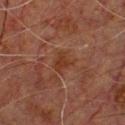Acquisition and patient details:
A male patient, aged around 50. From the chest. Imaged with cross-polarized lighting. The total-body-photography lesion software estimated internal color variation of about 1.5 on a 0–10 scale. Longest diameter approximately 2.5 mm. A 15 mm close-up extracted from a 3D total-body photography capture.